This image is a 15 mm lesion crop taken from a total-body photograph. Located on the back. The patient is a male aged approximately 75 — 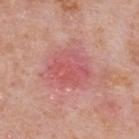Findings:
– image-analysis metrics · a lesion color around L≈57 a*≈32 b*≈24 in CIELAB, a lesion–skin lightness drop of about 8, and a lesion-to-skin contrast of about 5 (normalized; higher = more distinct)
– tile lighting · white-light illumination
– diameter · about 5 mm
– histopathologic diagnosis · a nodular basal cell carcinoma — a malignant lesion